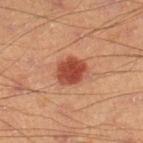Findings:
- biopsy status: catalogued during a skin exam; not biopsied